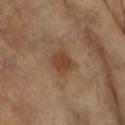Imaged during a routine full-body skin examination; the lesion was not biopsied and no histopathology is available. Imaged with cross-polarized lighting. Longest diameter approximately 3 mm. This image is a 15 mm lesion crop taken from a total-body photograph. The patient is a female aged 73–77. Located on the right forearm.